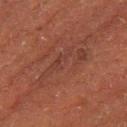{"biopsy_status": "not biopsied; imaged during a skin examination", "automated_metrics": {"nevus_likeness_0_100": 0, "lesion_detection_confidence_0_100": 65}, "image": {"source": "total-body photography crop", "field_of_view_mm": 15}, "site": "right thigh", "patient": {"sex": "male", "age_approx": 75}, "lighting": "cross-polarized"}